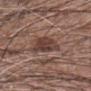Context:
A region of skin cropped from a whole-body photographic capture, roughly 15 mm wide. Automated image analysis of the tile measured a lesion area of about 8 mm², an eccentricity of roughly 0.7, and two-axis asymmetry of about 0.35. It also reported a lesion–skin lightness drop of about 11 and a normalized lesion–skin contrast near 9. And it measured border irregularity of about 3 on a 0–10 scale, a within-lesion color-variation index near 3.5/10, and a peripheral color-asymmetry measure near 1. The lesion is on the left forearm. Captured under white-light illumination. A male subject approximately 60 years of age. About 4 mm across.